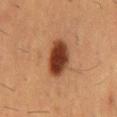Assessment:
The lesion was photographed on a routine skin check and not biopsied; there is no pathology result.
Background:
A male patient aged 53–57. A lesion tile, about 15 mm wide, cut from a 3D total-body photograph. Approximately 5 mm at its widest. Located on the abdomen.